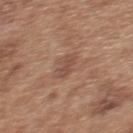biopsy_status: not biopsied; imaged during a skin examination
lesion_size:
  long_diameter_mm_approx: 2.5
automated_metrics:
  area_mm2_approx: 4.5
  shape_asymmetry: 0.2
  vs_skin_darker_L: 7.0
  nevus_likeness_0_100: 0
  lesion_detection_confidence_0_100: 100
patient:
  sex: female
  age_approx: 40
site: upper back
image:
  source: total-body photography crop
  field_of_view_mm: 15
lighting: white-light